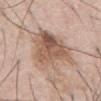Captured during whole-body skin photography for melanoma surveillance; the lesion was not biopsied.
The recorded lesion diameter is about 6.5 mm.
Cropped from a total-body skin-imaging series; the visible field is about 15 mm.
The lesion-visualizer software estimated an area of roughly 25 mm², a shape eccentricity near 0.6, and a shape-asymmetry score of about 0.4 (0 = symmetric). The analysis additionally found a lesion color around L≈57 a*≈18 b*≈28 in CIELAB and a lesion–skin lightness drop of about 12. The analysis additionally found a nevus-likeness score of about 15/100 and a lesion-detection confidence of about 100/100.
A male subject in their 70s.
From the chest.
This is a white-light tile.